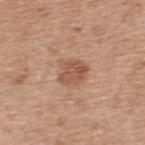{"patient": {"sex": "male", "age_approx": 55}, "automated_metrics": {"area_mm2_approx": 7.5, "eccentricity": 0.45, "shape_asymmetry": 0.25, "cielab_L": 54, "cielab_a": 22, "cielab_b": 31, "vs_skin_darker_L": 10.0, "vs_skin_contrast_norm": 7.0, "color_variation_0_10": 3.5, "peripheral_color_asymmetry": 1.5}, "site": "upper back", "image": {"source": "total-body photography crop", "field_of_view_mm": 15}}